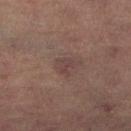Imaged during a routine full-body skin examination; the lesion was not biopsied and no histopathology is available. The lesion's longest dimension is about 2.5 mm. A lesion tile, about 15 mm wide, cut from a 3D total-body photograph. Located on the right lower leg. Captured under cross-polarized illumination. A female subject roughly 70 years of age.